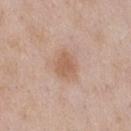The tile uses white-light illumination. Cropped from a whole-body photographic skin survey; the tile spans about 15 mm. The lesion is located on the front of the torso. Automated tile analysis of the lesion measured a mean CIELAB color near L≈59 a*≈19 b*≈31, a lesion–skin lightness drop of about 8, and a normalized lesion–skin contrast near 6.5. The patient is a male in their mid- to late 50s.